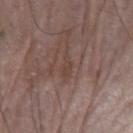Recorded during total-body skin imaging; not selected for excision or biopsy. Located on the left forearm. This is a white-light tile. A roughly 15 mm field-of-view crop from a total-body skin photograph. Automated image analysis of the tile measured border irregularity of about 4 on a 0–10 scale, internal color variation of about 0.5 on a 0–10 scale, and radial color variation of about 0. The analysis additionally found an automated nevus-likeness rating near 0 out of 100 and a detector confidence of about 70 out of 100 that the crop contains a lesion. A male subject in their mid- to late 60s.